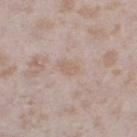notes: total-body-photography surveillance lesion; no biopsy | imaging modality: ~15 mm tile from a whole-body skin photo | patient: female, in their mid- to late 20s | lighting: white-light | body site: the right thigh.